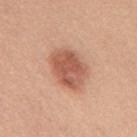Clinical impression: No biopsy was performed on this lesion — it was imaged during a full skin examination and was not determined to be concerning. Clinical summary: Measured at roughly 5 mm in maximum diameter. A female patient aged around 35. A region of skin cropped from a whole-body photographic capture, roughly 15 mm wide. The lesion is located on the mid back. Imaged with white-light lighting.